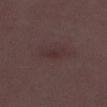Findings:
• follow-up: no biopsy performed (imaged during a skin exam)
• patient: female, aged approximately 30
• automated lesion analysis: a lesion area of about 4 mm², an eccentricity of roughly 0.95, and two-axis asymmetry of about 0.4; an average lesion color of about L≈30 a*≈17 b*≈15 (CIELAB), about 5 CIELAB-L* units darker than the surrounding skin, and a normalized lesion–skin contrast near 5.5; a nevus-likeness score of about 0/100 and a lesion-detection confidence of about 100/100
• anatomic site: the right thigh
• lesion diameter: about 3.5 mm
• image: ~15 mm crop, total-body skin-cancer survey